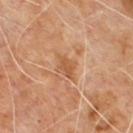Clinical summary:
An algorithmic analysis of the crop reported an area of roughly 4.5 mm², a shape eccentricity near 0.7, and a symmetry-axis asymmetry near 0.25. The software also gave a lesion color around L≈53 a*≈22 b*≈36 in CIELAB, a lesion–skin lightness drop of about 8, and a normalized lesion–skin contrast near 6.5. The software also gave an automated nevus-likeness rating near 0 out of 100 and a detector confidence of about 95 out of 100 that the crop contains a lesion. On the upper back. Captured under cross-polarized illumination. The lesion's longest dimension is about 3 mm. The patient is a male approximately 60 years of age. Cropped from a whole-body photographic skin survey; the tile spans about 15 mm.